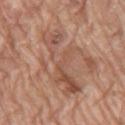Assessment: The lesion was tiled from a total-body skin photograph and was not biopsied. Image and clinical context: The lesion's longest dimension is about 8.5 mm. The lesion is on the right thigh. Captured under white-light illumination. A female subject, in their mid- to late 70s. A lesion tile, about 15 mm wide, cut from a 3D total-body photograph.